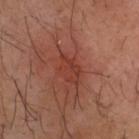Q: Was this lesion biopsied?
A: imaged on a skin check; not biopsied
Q: Automated lesion metrics?
A: a footprint of about 6.5 mm², a shape eccentricity near 0.75, and a symmetry-axis asymmetry near 0.2; an average lesion color of about L≈38 a*≈27 b*≈28 (CIELAB) and a lesion-to-skin contrast of about 5.5 (normalized; higher = more distinct); a border-irregularity index near 3/10, a within-lesion color-variation index near 3/10, and a peripheral color-asymmetry measure near 1
Q: How was this image acquired?
A: 15 mm crop, total-body photography
Q: What are the patient's age and sex?
A: male, roughly 40 years of age
Q: What is the lesion's diameter?
A: ~4 mm (longest diameter)
Q: How was the tile lit?
A: cross-polarized illumination
Q: Lesion location?
A: the head or neck